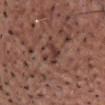Clinical impression: The lesion was photographed on a routine skin check and not biopsied; there is no pathology result. Context: The subject is a male aged around 80. Located on the chest. Approximately 3.5 mm at its widest. The tile uses white-light illumination. Cropped from a total-body skin-imaging series; the visible field is about 15 mm.